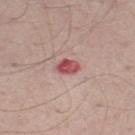follow-up = imaged on a skin check; not biopsied | diameter = ~2.5 mm (longest diameter) | automated lesion analysis = an area of roughly 4.5 mm², a shape eccentricity near 0.65, and a symmetry-axis asymmetry near 0.2 | image = total-body-photography crop, ~15 mm field of view | subject = male, aged 58–62 | site = the right thigh | lighting = white-light illumination.